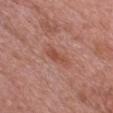No biopsy was performed on this lesion — it was imaged during a full skin examination and was not determined to be concerning. The lesion is on the chest. Imaged with white-light lighting. The subject is a female approximately 60 years of age. A 15 mm close-up extracted from a 3D total-body photography capture.